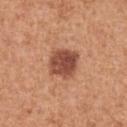The lesion was photographed on a routine skin check and not biopsied; there is no pathology result.